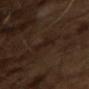<record>
<biopsy_status>not biopsied; imaged during a skin examination</biopsy_status>
<patient>
  <sex>male</sex>
  <age_approx>65</age_approx>
</patient>
<image>
  <source>total-body photography crop</source>
  <field_of_view_mm>15</field_of_view_mm>
</image>
</record>